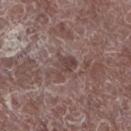Impression:
The lesion was photographed on a routine skin check and not biopsied; there is no pathology result.